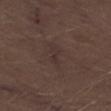Findings:
- image source: 15 mm crop, total-body photography
- site: the right thigh
- lesion diameter: about 3 mm
- subject: male, about 70 years old
- lighting: white-light illumination